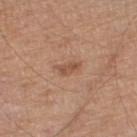Assessment: Part of a total-body skin-imaging series; this lesion was reviewed on a skin check and was not flagged for biopsy. Clinical summary: A male subject aged around 70. A lesion tile, about 15 mm wide, cut from a 3D total-body photograph. From the leg. Longest diameter approximately 2.5 mm. The lesion-visualizer software estimated an area of roughly 3 mm² and a symmetry-axis asymmetry near 0.3. And it measured a border-irregularity rating of about 3/10 and a peripheral color-asymmetry measure near 0.5. And it measured an automated nevus-likeness rating near 10 out of 100.